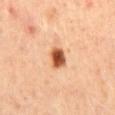notes: imaged on a skin check; not biopsied | imaging modality: ~15 mm tile from a whole-body skin photo | subject: female, aged 43–47 | image-analysis metrics: a shape eccentricity near 0.6 and a shape-asymmetry score of about 0.25 (0 = symmetric) | anatomic site: the mid back | diameter: ≈3 mm.